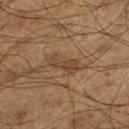Captured during whole-body skin photography for melanoma surveillance; the lesion was not biopsied. A 15 mm close-up extracted from a 3D total-body photography capture. The lesion is on the leg. The lesion's longest dimension is about 3.5 mm. A male subject roughly 60 years of age.